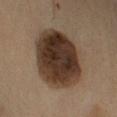biopsy status: total-body-photography surveillance lesion; no biopsy
subject: male, about 55 years old
TBP lesion metrics: a lesion area of about 40 mm², an eccentricity of roughly 0.5, and a shape-asymmetry score of about 0.1 (0 = symmetric); a border-irregularity index near 1.5/10, internal color variation of about 6.5 on a 0–10 scale, and radial color variation of about 2
acquisition: 15 mm crop, total-body photography
lighting: cross-polarized illumination
site: the left upper arm